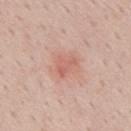Impression:
Part of a total-body skin-imaging series; this lesion was reviewed on a skin check and was not flagged for biopsy.
Clinical summary:
A male patient, roughly 50 years of age. The lesion is on the mid back. Cropped from a total-body skin-imaging series; the visible field is about 15 mm.